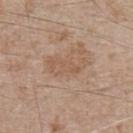Notes:
- workup: total-body-photography surveillance lesion; no biopsy
- patient: male, in their mid- to late 60s
- diameter: ~4.5 mm (longest diameter)
- image-analysis metrics: a border-irregularity index near 5/10, internal color variation of about 2 on a 0–10 scale, and a peripheral color-asymmetry measure near 0.5
- tile lighting: white-light illumination
- body site: the chest
- image: total-body-photography crop, ~15 mm field of view